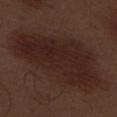follow-up=catalogued during a skin exam; not biopsied
body site=the left thigh
imaging modality=total-body-photography crop, ~15 mm field of view
subject=male, roughly 70 years of age